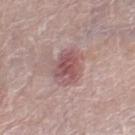Assessment: Captured during whole-body skin photography for melanoma surveillance; the lesion was not biopsied. Context: Automated image analysis of the tile measured a border-irregularity rating of about 3/10, a color-variation rating of about 4.5/10, and radial color variation of about 1.5. A roughly 15 mm field-of-view crop from a total-body skin photograph. The patient is a male approximately 80 years of age. From the right thigh.